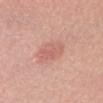| field | value |
|---|---|
| notes | total-body-photography surveillance lesion; no biopsy |
| location | the head or neck |
| subject | female, aged 53 to 57 |
| imaging modality | 15 mm crop, total-body photography |